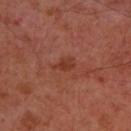follow-up = imaged on a skin check; not biopsied
acquisition = 15 mm crop, total-body photography
location = the upper back
patient = male, aged around 50
size = ~2.5 mm (longest diameter)